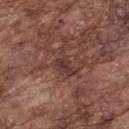Assessment: This lesion was catalogued during total-body skin photography and was not selected for biopsy. Acquisition and patient details: The lesion-visualizer software estimated border irregularity of about 6.5 on a 0–10 scale. And it measured a classifier nevus-likeness of about 0/100 and a detector confidence of about 90 out of 100 that the crop contains a lesion. About 4 mm across. A male subject in their mid- to late 70s. From the upper back. The tile uses white-light illumination. A region of skin cropped from a whole-body photographic capture, roughly 15 mm wide.